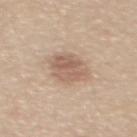This lesion was catalogued during total-body skin photography and was not selected for biopsy. The total-body-photography lesion software estimated a mean CIELAB color near L≈60 a*≈17 b*≈28, roughly 10 lightness units darker than nearby skin, and a lesion-to-skin contrast of about 6.5 (normalized; higher = more distinct). About 4.5 mm across. The patient is a female aged around 45. Captured under white-light illumination. On the back. Cropped from a whole-body photographic skin survey; the tile spans about 15 mm.